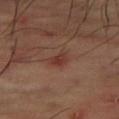workup = catalogued during a skin exam; not biopsied | subject = male, roughly 65 years of age | site = the right thigh | imaging modality = 15 mm crop, total-body photography | tile lighting = cross-polarized illumination.